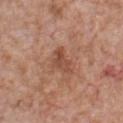Case summary:
• workup — total-body-photography surveillance lesion; no biopsy
• anatomic site — the chest
• subject — male, roughly 65 years of age
• tile lighting — white-light illumination
• size — ~3.5 mm (longest diameter)
• image source — 15 mm crop, total-body photography
• TBP lesion metrics — a lesion area of about 5 mm², an outline eccentricity of about 0.8 (0 = round, 1 = elongated), and two-axis asymmetry of about 0.5; an average lesion color of about L≈49 a*≈23 b*≈31 (CIELAB), about 9 CIELAB-L* units darker than the surrounding skin, and a normalized lesion–skin contrast near 6.5; border irregularity of about 5 on a 0–10 scale, a within-lesion color-variation index near 2/10, and peripheral color asymmetry of about 1; an automated nevus-likeness rating near 0 out of 100